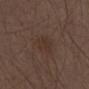{"biopsy_status": "not biopsied; imaged during a skin examination", "lesion_size": {"long_diameter_mm_approx": 4.0}, "image": {"source": "total-body photography crop", "field_of_view_mm": 15}, "patient": {"sex": "male", "age_approx": 70}, "site": "abdomen"}